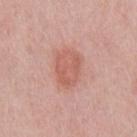follow-up: no biopsy performed (imaged during a skin exam) | automated lesion analysis: a footprint of about 11 mm² and a symmetry-axis asymmetry near 0.2; an average lesion color of about L≈59 a*≈26 b*≈28 (CIELAB), a lesion–skin lightness drop of about 8, and a lesion-to-skin contrast of about 6 (normalized; higher = more distinct); a nevus-likeness score of about 70/100 and a lesion-detection confidence of about 100/100 | subject: male, about 50 years old | acquisition: 15 mm crop, total-body photography | lesion size: ≈4 mm | body site: the front of the torso.